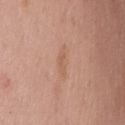<record>
  <biopsy_status>not biopsied; imaged during a skin examination</biopsy_status>
  <patient>
    <sex>male</sex>
    <age_approx>55</age_approx>
  </patient>
  <image>
    <source>total-body photography crop</source>
    <field_of_view_mm>15</field_of_view_mm>
  </image>
  <site>front of the torso</site>
</record>